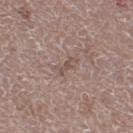Clinical impression:
Captured during whole-body skin photography for melanoma surveillance; the lesion was not biopsied.
Background:
A female patient in their mid-60s. The lesion is located on the right thigh. The lesion's longest dimension is about 2.5 mm. A roughly 15 mm field-of-view crop from a total-body skin photograph. Captured under white-light illumination.